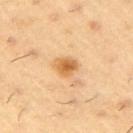Clinical impression: Recorded during total-body skin imaging; not selected for excision or biopsy. Background: Captured under cross-polarized illumination. From the right upper arm. A male subject, roughly 55 years of age. About 2.5 mm across. A region of skin cropped from a whole-body photographic capture, roughly 15 mm wide.